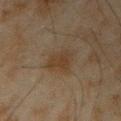Recorded during total-body skin imaging; not selected for excision or biopsy.
A close-up tile cropped from a whole-body skin photograph, about 15 mm across.
The lesion is on the left forearm.
This is a cross-polarized tile.
The recorded lesion diameter is about 3.5 mm.
A male subject, aged approximately 45.